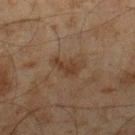No biopsy was performed on this lesion — it was imaged during a full skin examination and was not determined to be concerning. Automated image analysis of the tile measured a lesion area of about 4.5 mm² and two-axis asymmetry of about 0.4. And it measured an average lesion color of about L≈30 a*≈14 b*≈24 (CIELAB), roughly 6 lightness units darker than nearby skin, and a normalized border contrast of about 7. And it measured an automated nevus-likeness rating near 0 out of 100. This is a cross-polarized tile. A male patient, in their mid- to late 40s. From the leg. A 15 mm close-up extracted from a 3D total-body photography capture. Measured at roughly 3 mm in maximum diameter.